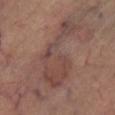Impression:
Part of a total-body skin-imaging series; this lesion was reviewed on a skin check and was not flagged for biopsy.
Acquisition and patient details:
The lesion is located on the right lower leg. The tile uses cross-polarized illumination. A region of skin cropped from a whole-body photographic capture, roughly 15 mm wide. A female subject aged 63–67.